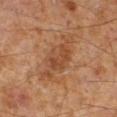Impression:
Part of a total-body skin-imaging series; this lesion was reviewed on a skin check and was not flagged for biopsy.
Image and clinical context:
About 6.5 mm across. The patient is a male roughly 60 years of age. Located on the right lower leg. A 15 mm close-up tile from a total-body photography series done for melanoma screening. The tile uses cross-polarized illumination.